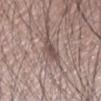Captured during whole-body skin photography for melanoma surveillance; the lesion was not biopsied.
This is a white-light tile.
The patient is a male roughly 50 years of age.
From the right forearm.
This image is a 15 mm lesion crop taken from a total-body photograph.
Approximately 3 mm at its widest.
Automated image analysis of the tile measured a lesion area of about 5 mm², an eccentricity of roughly 0.8, and a symmetry-axis asymmetry near 0.35. The software also gave a border-irregularity rating of about 3.5/10, a color-variation rating of about 2.5/10, and radial color variation of about 1. The software also gave a classifier nevus-likeness of about 5/100 and lesion-presence confidence of about 85/100.